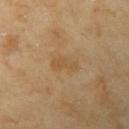notes: catalogued during a skin exam; not biopsied
lesion diameter: ≈3.5 mm
subject: female, roughly 70 years of age
tile lighting: cross-polarized
imaging modality: ~15 mm crop, total-body skin-cancer survey
location: the left upper arm